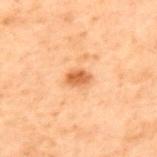notes: total-body-photography surveillance lesion; no biopsy | illumination: cross-polarized illumination | imaging modality: ~15 mm crop, total-body skin-cancer survey | anatomic site: the upper back | TBP lesion metrics: a border-irregularity index near 2/10, internal color variation of about 2.5 on a 0–10 scale, and radial color variation of about 1; a classifier nevus-likeness of about 95/100 and a lesion-detection confidence of about 100/100 | patient: male, aged 68–72 | lesion diameter: about 2.5 mm.